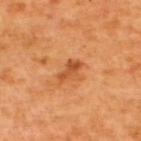Captured during whole-body skin photography for melanoma surveillance; the lesion was not biopsied. Cropped from a total-body skin-imaging series; the visible field is about 15 mm. The recorded lesion diameter is about 3 mm. The lesion is on the upper back. A male subject aged approximately 65.